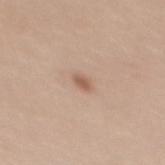| field | value |
|---|---|
| workup | no biopsy performed (imaged during a skin exam) |
| image source | total-body-photography crop, ~15 mm field of view |
| lesion size | ~2 mm (longest diameter) |
| site | the upper back |
| subject | female, aged 33–37 |
| tile lighting | white-light |